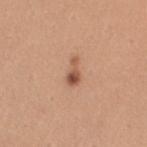This lesion was catalogued during total-body skin photography and was not selected for biopsy. The patient is a female in their 30s. Captured under white-light illumination. Located on the left upper arm. Measured at roughly 3 mm in maximum diameter. A region of skin cropped from a whole-body photographic capture, roughly 15 mm wide.